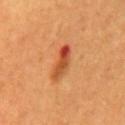Impression: The lesion was tiled from a total-body skin photograph and was not biopsied. Context: From the mid back. A male patient roughly 60 years of age. Measured at roughly 4.5 mm in maximum diameter. Automated tile analysis of the lesion measured an outline eccentricity of about 0.9 (0 = round, 1 = elongated) and a symmetry-axis asymmetry near 0.25. And it measured border irregularity of about 3 on a 0–10 scale and a peripheral color-asymmetry measure near 5. The software also gave a lesion-detection confidence of about 100/100. A region of skin cropped from a whole-body photographic capture, roughly 15 mm wide.